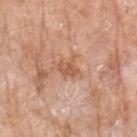- biopsy status — catalogued during a skin exam; not biopsied
- acquisition — total-body-photography crop, ~15 mm field of view
- patient — female, in their mid-70s
- tile lighting — white-light
- diameter — ~2.5 mm (longest diameter)
- location — the left forearm